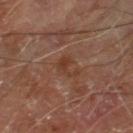The lesion-visualizer software estimated a shape-asymmetry score of about 0.55 (0 = symmetric). The analysis additionally found an average lesion color of about L≈37 a*≈21 b*≈28 (CIELAB), a lesion–skin lightness drop of about 6, and a normalized lesion–skin contrast near 6.5. And it measured a classifier nevus-likeness of about 0/100 and lesion-presence confidence of about 100/100. Longest diameter approximately 3 mm. The lesion is located on the right lower leg. The subject is a male in their 70s. Cropped from a total-body skin-imaging series; the visible field is about 15 mm.